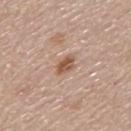The lesion was tiled from a total-body skin photograph and was not biopsied.